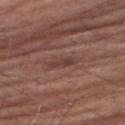Notes:
– workup — imaged on a skin check; not biopsied
– size — ≈3.5 mm
– imaging modality — ~15 mm tile from a whole-body skin photo
– subject — male, roughly 85 years of age or older
– anatomic site — the right upper arm